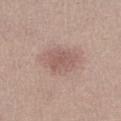Imaged during a routine full-body skin examination; the lesion was not biopsied and no histopathology is available.
From the right lower leg.
Imaged with white-light lighting.
A female patient, aged 18–22.
Cropped from a whole-body photographic skin survey; the tile spans about 15 mm.